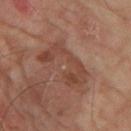Q: Was a biopsy performed?
A: no biopsy performed (imaged during a skin exam)
Q: How was the tile lit?
A: cross-polarized
Q: Automated lesion metrics?
A: a footprint of about 13 mm², a shape eccentricity near 0.85, and a symmetry-axis asymmetry near 0.5; about 7 CIELAB-L* units darker than the surrounding skin and a normalized lesion–skin contrast near 6; a border-irregularity rating of about 9/10, a within-lesion color-variation index near 4/10, and a peripheral color-asymmetry measure near 1.5; a classifier nevus-likeness of about 0/100 and a lesion-detection confidence of about 100/100
Q: What is the imaging modality?
A: 15 mm crop, total-body photography
Q: Lesion size?
A: about 6 mm
Q: What are the patient's age and sex?
A: male, in their mid- to late 60s
Q: Lesion location?
A: the left thigh